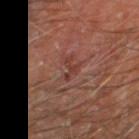Impression: Imaged during a routine full-body skin examination; the lesion was not biopsied and no histopathology is available. Clinical summary: Cropped from a whole-body photographic skin survey; the tile spans about 15 mm. The lesion is located on the right thigh. This is a cross-polarized tile. A male patient aged around 70. Approximately 3 mm at its widest.